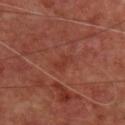Q: Is there a histopathology result?
A: catalogued during a skin exam; not biopsied
Q: What are the patient's age and sex?
A: female, in their mid- to late 40s
Q: What is the imaging modality?
A: total-body-photography crop, ~15 mm field of view
Q: Lesion size?
A: about 2.5 mm
Q: Illumination type?
A: cross-polarized
Q: Automated lesion metrics?
A: an outline eccentricity of about 0.85 (0 = round, 1 = elongated) and a symmetry-axis asymmetry near 0.4; about 5 CIELAB-L* units darker than the surrounding skin and a normalized border contrast of about 4.5; a border-irregularity rating of about 4/10, a within-lesion color-variation index near 0/10, and radial color variation of about 0; an automated nevus-likeness rating near 0 out of 100 and a lesion-detection confidence of about 100/100
Q: Lesion location?
A: the back